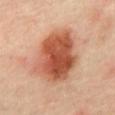biopsy status: catalogued during a skin exam; not biopsied | site: the abdomen | size: about 7 mm | image: total-body-photography crop, ~15 mm field of view | subject: male, in their mid-60s.